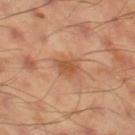<record>
<biopsy_status>not biopsied; imaged during a skin examination</biopsy_status>
<lighting>cross-polarized</lighting>
<lesion_size>
  <long_diameter_mm_approx>3.0</long_diameter_mm_approx>
</lesion_size>
<image>
  <source>total-body photography crop</source>
  <field_of_view_mm>15</field_of_view_mm>
</image>
<site>leg</site>
<patient>
  <sex>male</sex>
  <age_approx>45</age_approx>
</patient>
<automated_metrics>
  <area_mm2_approx>5.0</area_mm2_approx>
  <shape_asymmetry>0.3</shape_asymmetry>
  <nevus_likeness_0_100>60</nevus_likeness_0_100>
  <lesion_detection_confidence_0_100>100</lesion_detection_confidence_0_100>
</automated_metrics>
</record>